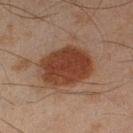This is a cross-polarized tile. Approximately 6 mm at its widest. A male subject, aged around 45. A 15 mm close-up tile from a total-body photography series done for melanoma screening. Located on the left lower leg. An algorithmic analysis of the crop reported an area of roughly 25 mm², an outline eccentricity of about 0.65 (0 = round, 1 = elongated), and a symmetry-axis asymmetry near 0.1. The analysis additionally found an average lesion color of about L≈32 a*≈18 b*≈25 (CIELAB), roughly 11 lightness units darker than nearby skin, and a lesion-to-skin contrast of about 10.5 (normalized; higher = more distinct). It also reported a border-irregularity index near 1/10 and a within-lesion color-variation index near 3.5/10.